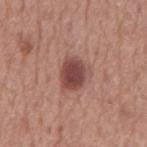{
  "biopsy_status": "not biopsied; imaged during a skin examination",
  "image": {
    "source": "total-body photography crop",
    "field_of_view_mm": 15
  },
  "site": "mid back",
  "patient": {
    "sex": "male",
    "age_approx": 75
  }
}